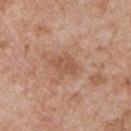Assessment:
The lesion was photographed on a routine skin check and not biopsied; there is no pathology result.
Context:
The subject is a male roughly 65 years of age. An algorithmic analysis of the crop reported an average lesion color of about L≈54 a*≈21 b*≈31 (CIELAB), roughly 8 lightness units darker than nearby skin, and a lesion-to-skin contrast of about 6 (normalized; higher = more distinct). It also reported a color-variation rating of about 2/10 and peripheral color asymmetry of about 0.5. The analysis additionally found an automated nevus-likeness rating near 0 out of 100 and lesion-presence confidence of about 100/100. Captured under white-light illumination. Cropped from a total-body skin-imaging series; the visible field is about 15 mm. Located on the chest.